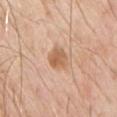| feature | finding |
|---|---|
| notes | imaged on a skin check; not biopsied |
| body site | the front of the torso |
| lighting | white-light |
| acquisition | 15 mm crop, total-body photography |
| size | ≈3 mm |
| subject | male, in their mid- to late 60s |